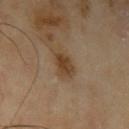| key | value |
|---|---|
| workup | imaged on a skin check; not biopsied |
| size | ≈3 mm |
| patient | male, aged 63–67 |
| site | the left upper arm |
| image source | ~15 mm tile from a whole-body skin photo |